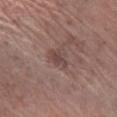{
  "biopsy_status": "not biopsied; imaged during a skin examination",
  "image": {
    "source": "total-body photography crop",
    "field_of_view_mm": 15
  },
  "site": "right lower leg",
  "patient": {
    "sex": "male",
    "age_approx": 70
  }
}